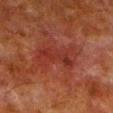Clinical impression: Part of a total-body skin-imaging series; this lesion was reviewed on a skin check and was not flagged for biopsy. Image and clinical context: The recorded lesion diameter is about 4.5 mm. The total-body-photography lesion software estimated a footprint of about 5.5 mm² and a shape-asymmetry score of about 0.45 (0 = symmetric). The analysis additionally found an average lesion color of about L≈26 a*≈26 b*≈25 (CIELAB), about 5 CIELAB-L* units darker than the surrounding skin, and a normalized border contrast of about 6. A lesion tile, about 15 mm wide, cut from a 3D total-body photograph. Captured under cross-polarized illumination. Located on the leg. The patient is a male roughly 80 years of age.